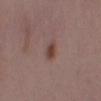The lesion was tiled from a total-body skin photograph and was not biopsied. The lesion is on the mid back. About 3 mm across. A close-up tile cropped from a whole-body skin photograph, about 15 mm across. This is a white-light tile. Automated image analysis of the tile measured a lesion area of about 4 mm², an outline eccentricity of about 0.85 (0 = round, 1 = elongated), and two-axis asymmetry of about 0.25. The analysis additionally found a lesion-detection confidence of about 100/100. A female subject, aged around 35.